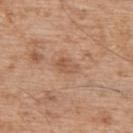Impression: Captured during whole-body skin photography for melanoma surveillance; the lesion was not biopsied. Clinical summary: The patient is a male approximately 55 years of age. On the upper back. This is a white-light tile. Cropped from a total-body skin-imaging series; the visible field is about 15 mm. Approximately 2.5 mm at its widest.